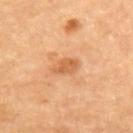A roughly 15 mm field-of-view crop from a total-body skin photograph.
An algorithmic analysis of the crop reported an area of roughly 4 mm², a shape eccentricity near 0.75, and a symmetry-axis asymmetry near 0.25. The software also gave a within-lesion color-variation index near 1.5/10 and a peripheral color-asymmetry measure near 0.5. And it measured a nevus-likeness score of about 0/100 and lesion-presence confidence of about 100/100.
The tile uses cross-polarized illumination.
The lesion is located on the left upper arm.
A male subject, aged approximately 85 or older.